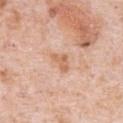| key | value |
|---|---|
| workup | no biopsy performed (imaged during a skin exam) |
| tile lighting | white-light illumination |
| TBP lesion metrics | a border-irregularity index near 4.5/10, a color-variation rating of about 2/10, and peripheral color asymmetry of about 0.5; lesion-presence confidence of about 100/100 |
| image | ~15 mm tile from a whole-body skin photo |
| patient | male, aged 78–82 |
| body site | the chest |